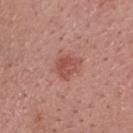Assessment:
Imaged during a routine full-body skin examination; the lesion was not biopsied and no histopathology is available.
Clinical summary:
The lesion is located on the head or neck. The lesion's longest dimension is about 3 mm. This is a white-light tile. A male subject, approximately 40 years of age. A 15 mm crop from a total-body photograph taken for skin-cancer surveillance.